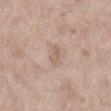No biopsy was performed on this lesion — it was imaged during a full skin examination and was not determined to be concerning.
From the right lower leg.
The lesion's longest dimension is about 3 mm.
This image is a 15 mm lesion crop taken from a total-body photograph.
A female subject, about 75 years old.